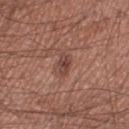The lesion was photographed on a routine skin check and not biopsied; there is no pathology result. Longest diameter approximately 3 mm. A male patient aged 53 to 57. This is a white-light tile. From the right thigh. An algorithmic analysis of the crop reported a nevus-likeness score of about 40/100 and a lesion-detection confidence of about 100/100. A close-up tile cropped from a whole-body skin photograph, about 15 mm across.